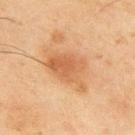No biopsy was performed on this lesion — it was imaged during a full skin examination and was not determined to be concerning. On the back. An algorithmic analysis of the crop reported a footprint of about 15 mm², a shape eccentricity near 0.75, and a symmetry-axis asymmetry near 0.35. And it measured a border-irregularity index near 4.5/10 and internal color variation of about 3.5 on a 0–10 scale. This is a cross-polarized tile. A male patient, about 45 years old. Cropped from a whole-body photographic skin survey; the tile spans about 15 mm.